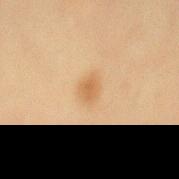Assessment: This lesion was catalogued during total-body skin photography and was not selected for biopsy. Background: This is a cross-polarized tile. A male patient roughly 60 years of age. The lesion is on the mid back. Longest diameter approximately 2.5 mm. A close-up tile cropped from a whole-body skin photograph, about 15 mm across.